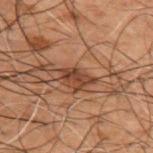biopsy status: total-body-photography surveillance lesion; no biopsy | subject: male, approximately 50 years of age | site: the upper back | lesion diameter: ~3.5 mm (longest diameter) | image source: 15 mm crop, total-body photography.